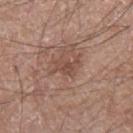workup = imaged on a skin check; not biopsied